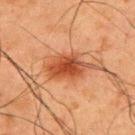This lesion was catalogued during total-body skin photography and was not selected for biopsy. The recorded lesion diameter is about 5.5 mm. From the back. The tile uses cross-polarized illumination. The patient is a male approximately 60 years of age. A lesion tile, about 15 mm wide, cut from a 3D total-body photograph.